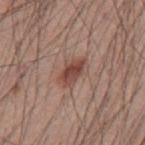follow-up: no biopsy performed (imaged during a skin exam)
image: ~15 mm crop, total-body skin-cancer survey
automated metrics: an area of roughly 7 mm² and a shape-asymmetry score of about 0.25 (0 = symmetric); a lesion color around L≈46 a*≈22 b*≈25 in CIELAB, a lesion–skin lightness drop of about 11, and a lesion-to-skin contrast of about 8 (normalized; higher = more distinct); a border-irregularity index near 3/10, a within-lesion color-variation index near 4.5/10, and a peripheral color-asymmetry measure near 1.5
anatomic site: the mid back
lighting: white-light illumination
subject: male, in their 60s
lesion diameter: ≈4 mm